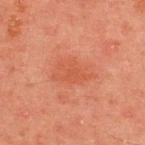workup=imaged on a skin check; not biopsied
imaging modality=total-body-photography crop, ~15 mm field of view
lighting=cross-polarized
location=the upper back
patient=male, approximately 45 years of age
diameter=~5 mm (longest diameter)